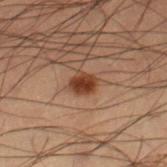Recorded during total-body skin imaging; not selected for excision or biopsy. The patient is a male aged approximately 55. A close-up tile cropped from a whole-body skin photograph, about 15 mm across. On the right lower leg.